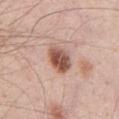{
  "image": {
    "source": "total-body photography crop",
    "field_of_view_mm": 15
  },
  "patient": {
    "sex": "male",
    "age_approx": 40
  },
  "lighting": "white-light",
  "lesion_size": {
    "long_diameter_mm_approx": 3.5
  },
  "site": "chest",
  "automated_metrics": {
    "area_mm2_approx": 7.0,
    "eccentricity": 0.75,
    "shape_asymmetry": 0.15,
    "cielab_L": 53,
    "cielab_a": 23,
    "cielab_b": 27,
    "vs_skin_darker_L": 17.0,
    "vs_skin_contrast_norm": 11.0
  }
}